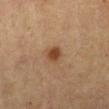A male patient about 60 years old. A 15 mm close-up extracted from a 3D total-body photography capture. The lesion is on the chest. Imaged with cross-polarized lighting.